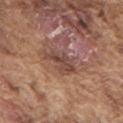Q: Is there a histopathology result?
A: no biopsy performed (imaged during a skin exam)